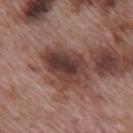Acquisition and patient details:
The lesion is on the mid back. The lesion-visualizer software estimated about 13 CIELAB-L* units darker than the surrounding skin and a normalized lesion–skin contrast near 10.5. The recorded lesion diameter is about 5.5 mm. A 15 mm close-up extracted from a 3D total-body photography capture. Captured under white-light illumination. A male patient aged approximately 70.